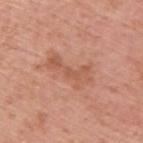This lesion was catalogued during total-body skin photography and was not selected for biopsy. About 5.5 mm across. A lesion tile, about 15 mm wide, cut from a 3D total-body photograph. Automated tile analysis of the lesion measured an average lesion color of about L≈56 a*≈25 b*≈32 (CIELAB), about 8 CIELAB-L* units darker than the surrounding skin, and a lesion-to-skin contrast of about 5.5 (normalized; higher = more distinct). It also reported a border-irregularity rating of about 7/10, a color-variation rating of about 2.5/10, and peripheral color asymmetry of about 0.5. The tile uses white-light illumination. A male subject, roughly 55 years of age. The lesion is on the upper back.